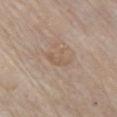Q: What kind of image is this?
A: total-body-photography crop, ~15 mm field of view
Q: What is the anatomic site?
A: the front of the torso
Q: Who is the patient?
A: male, aged 83 or older